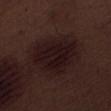workup = imaged on a skin check; not biopsied | anatomic site = the right thigh | patient = male, aged approximately 70 | tile lighting = white-light | size = ≈7 mm | acquisition = ~15 mm tile from a whole-body skin photo.